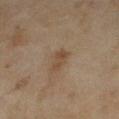The lesion was tiled from a total-body skin photograph and was not biopsied. Cropped from a total-body skin-imaging series; the visible field is about 15 mm. The recorded lesion diameter is about 3 mm. The lesion is located on the left lower leg. Imaged with cross-polarized lighting. The patient is a female approximately 60 years of age.